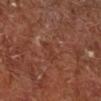Imaged during a routine full-body skin examination; the lesion was not biopsied and no histopathology is available.
The total-body-photography lesion software estimated a lesion area of about 2.5 mm², a shape eccentricity near 0.95, and two-axis asymmetry of about 0.4. The analysis additionally found an average lesion color of about L≈34 a*≈23 b*≈28 (CIELAB) and about 5 CIELAB-L* units darker than the surrounding skin. The analysis additionally found a border-irregularity index near 4.5/10, internal color variation of about 0 on a 0–10 scale, and a peripheral color-asymmetry measure near 0. The analysis additionally found an automated nevus-likeness rating near 0 out of 100 and lesion-presence confidence of about 95/100.
A male subject, aged around 65.
About 2.5 mm across.
This image is a 15 mm lesion crop taken from a total-body photograph.
This is a cross-polarized tile.
From the right lower leg.